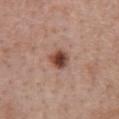biopsy status = catalogued during a skin exam; not biopsied
image-analysis metrics = a color-variation rating of about 8.5/10 and peripheral color asymmetry of about 3; a classifier nevus-likeness of about 100/100
location = the chest
lighting = white-light illumination
patient = female, aged approximately 45
diameter = about 2.5 mm
image = ~15 mm tile from a whole-body skin photo